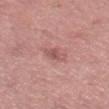Case summary:
• anatomic site — the left thigh
• image — 15 mm crop, total-body photography
• subject — male, roughly 50 years of age
• lesion size — about 3 mm
• lighting — white-light illumination